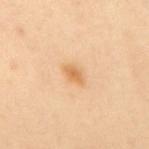{
  "biopsy_status": "not biopsied; imaged during a skin examination",
  "patient": {
    "sex": "female",
    "age_approx": 45
  },
  "lesion_size": {
    "long_diameter_mm_approx": 3.0
  },
  "site": "upper back",
  "lighting": "cross-polarized",
  "image": {
    "source": "total-body photography crop",
    "field_of_view_mm": 15
  }
}